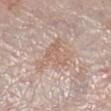biopsy_status: not biopsied; imaged during a skin examination